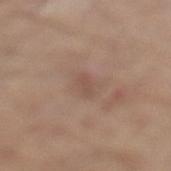biopsy status = no biopsy performed (imaged during a skin exam)
image source = ~15 mm crop, total-body skin-cancer survey
lighting = white-light
size = about 2.5 mm
subject = male, aged approximately 65
site = the left lower leg